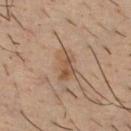Recorded during total-body skin imaging; not selected for excision or biopsy. A male subject, in their mid- to late 30s. The lesion is located on the chest. Captured under cross-polarized illumination. The lesion-visualizer software estimated a border-irregularity rating of about 2.5/10, a within-lesion color-variation index near 4.5/10, and a peripheral color-asymmetry measure near 1.5. The software also gave a nevus-likeness score of about 60/100 and a detector confidence of about 100 out of 100 that the crop contains a lesion. A 15 mm close-up extracted from a 3D total-body photography capture.